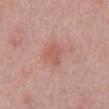workup: no biopsy performed (imaged during a skin exam) | subject: male, approximately 50 years of age | body site: the abdomen | image: ~15 mm tile from a whole-body skin photo.